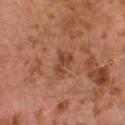workup=imaged on a skin check; not biopsied | patient=male, aged approximately 30 | lesion diameter=~3 mm (longest diameter) | image source=~15 mm crop, total-body skin-cancer survey | lighting=cross-polarized illumination | site=the left lower leg.